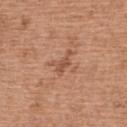Recorded during total-body skin imaging; not selected for excision or biopsy. The lesion-visualizer software estimated a lesion color around L≈53 a*≈23 b*≈32 in CIELAB, about 8 CIELAB-L* units darker than the surrounding skin, and a normalized border contrast of about 6. The analysis additionally found an automated nevus-likeness rating near 0 out of 100 and a detector confidence of about 100 out of 100 that the crop contains a lesion. This is a white-light tile. A female patient aged around 60. A 15 mm crop from a total-body photograph taken for skin-cancer surveillance. About 3.5 mm across. The lesion is located on the back.